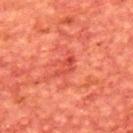This lesion was catalogued during total-body skin photography and was not selected for biopsy.